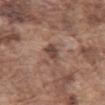notes: imaged on a skin check; not biopsied | automated lesion analysis: a lesion area of about 4 mm², an outline eccentricity of about 0.75 (0 = round, 1 = elongated), and a shape-asymmetry score of about 0.35 (0 = symmetric); a lesion color around L≈45 a*≈17 b*≈23 in CIELAB and a lesion–skin lightness drop of about 11; a border-irregularity rating of about 3/10 and peripheral color asymmetry of about 1 | location: the abdomen | subject: male, in their mid- to late 70s | illumination: white-light illumination | image source: 15 mm crop, total-body photography | diameter: ~3 mm (longest diameter).